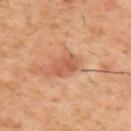Notes:
• biopsy status — catalogued during a skin exam; not biopsied
• subject — male, roughly 60 years of age
• body site — the upper back
• lesion diameter — about 3.5 mm
• image-analysis metrics — an automated nevus-likeness rating near 0 out of 100
• acquisition — 15 mm crop, total-body photography